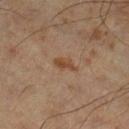Case summary:
* notes · total-body-photography surveillance lesion; no biopsy